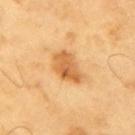notes = total-body-photography surveillance lesion; no biopsy
patient = male, roughly 60 years of age
acquisition = ~15 mm tile from a whole-body skin photo
site = the right upper arm